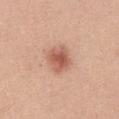Q: Is there a histopathology result?
A: no biopsy performed (imaged during a skin exam)
Q: Illumination type?
A: white-light
Q: How large is the lesion?
A: ≈3 mm
Q: Where on the body is the lesion?
A: the abdomen
Q: What did automated image analysis measure?
A: a lesion area of about 7.5 mm² and an eccentricity of roughly 0.45; border irregularity of about 2 on a 0–10 scale and a peripheral color-asymmetry measure near 1
Q: What kind of image is this?
A: ~15 mm crop, total-body skin-cancer survey
Q: What are the patient's age and sex?
A: female, approximately 35 years of age